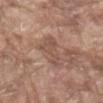Findings:
• biopsy status · no biopsy performed (imaged during a skin exam)
• body site · the abdomen
• patient · male, in their 80s
• image source · 15 mm crop, total-body photography